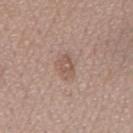Image and clinical context: The lesion is on the mid back. Cropped from a whole-body photographic skin survey; the tile spans about 15 mm. Automated image analysis of the tile measured a mean CIELAB color near L≈54 a*≈18 b*≈25, a lesion–skin lightness drop of about 8, and a lesion-to-skin contrast of about 5.5 (normalized; higher = more distinct). And it measured a border-irregularity index near 3/10, a color-variation rating of about 2.5/10, and radial color variation of about 1. About 3 mm across. A male subject, aged around 70.